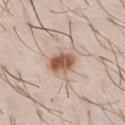Imaged during a routine full-body skin examination; the lesion was not biopsied and no histopathology is available. Approximately 3.5 mm at its widest. The patient is a male aged around 30. A close-up tile cropped from a whole-body skin photograph, about 15 mm across. From the chest.